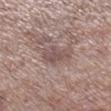Case summary:
• follow-up — total-body-photography surveillance lesion; no biopsy
• subject — male, approximately 55 years of age
• anatomic site — the right lower leg
• image — total-body-photography crop, ~15 mm field of view
• lesion diameter — ~3 mm (longest diameter)
• lighting — white-light
• image-analysis metrics — a lesion area of about 5 mm², an eccentricity of roughly 0.75, and a shape-asymmetry score of about 0.4 (0 = symmetric); an automated nevus-likeness rating near 0 out of 100 and a lesion-detection confidence of about 80/100